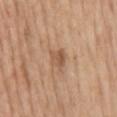The lesion was photographed on a routine skin check and not biopsied; there is no pathology result. The lesion is located on the mid back. Measured at roughly 3 mm in maximum diameter. A female patient roughly 65 years of age. Cropped from a total-body skin-imaging series; the visible field is about 15 mm.